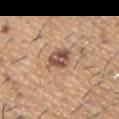Recorded during total-body skin imaging; not selected for excision or biopsy.
Captured under white-light illumination.
From the arm.
A male patient, about 60 years old.
The recorded lesion diameter is about 3.5 mm.
A roughly 15 mm field-of-view crop from a total-body skin photograph.